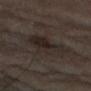Captured during whole-body skin photography for melanoma surveillance; the lesion was not biopsied.
Captured under cross-polarized illumination.
The recorded lesion diameter is about 5.5 mm.
On the left thigh.
This image is a 15 mm lesion crop taken from a total-body photograph.
A male subject, aged 83 or older.